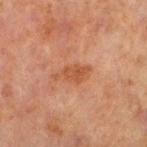{
  "biopsy_status": "not biopsied; imaged during a skin examination",
  "patient": {
    "sex": "male",
    "age_approx": 70
  },
  "lesion_size": {
    "long_diameter_mm_approx": 4.0
  },
  "image": {
    "source": "total-body photography crop",
    "field_of_view_mm": 15
  },
  "site": "left lower leg",
  "lighting": "cross-polarized"
}